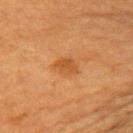The lesion was photographed on a routine skin check and not biopsied; there is no pathology result. On the right upper arm. Automated image analysis of the tile measured an outline eccentricity of about 0.65 (0 = round, 1 = elongated) and two-axis asymmetry of about 0.3. And it measured an average lesion color of about L≈43 a*≈23 b*≈37 (CIELAB) and about 7 CIELAB-L* units darker than the surrounding skin. The software also gave border irregularity of about 3 on a 0–10 scale, internal color variation of about 1.5 on a 0–10 scale, and a peripheral color-asymmetry measure near 0.5. The software also gave an automated nevus-likeness rating near 50 out of 100 and a detector confidence of about 100 out of 100 that the crop contains a lesion. The tile uses cross-polarized illumination. A 15 mm close-up tile from a total-body photography series done for melanoma screening. A female subject, in their mid- to late 50s. Approximately 3 mm at its widest.